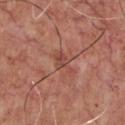Imaged during a routine full-body skin examination; the lesion was not biopsied and no histopathology is available. The lesion is located on the chest. Captured under white-light illumination. Approximately 3 mm at its widest. Cropped from a total-body skin-imaging series; the visible field is about 15 mm. A male patient, aged 63 to 67.